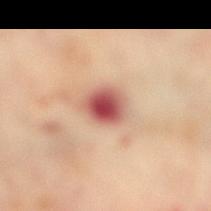Findings:
- body site — the right lower leg
- automated metrics — a footprint of about 8 mm², an eccentricity of roughly 0.55, and a symmetry-axis asymmetry near 0.2; a detector confidence of about 100 out of 100 that the crop contains a lesion
- lesion size — about 3.5 mm
- patient — female, aged around 65
- image source — total-body-photography crop, ~15 mm field of view
- illumination — cross-polarized